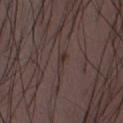The lesion was tiled from a total-body skin photograph and was not biopsied. A male patient aged 48 to 52. On the abdomen. A close-up tile cropped from a whole-body skin photograph, about 15 mm across. The tile uses white-light illumination.